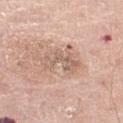Findings:
* notes — no biopsy performed (imaged during a skin exam)
* lighting — white-light
* acquisition — ~15 mm tile from a whole-body skin photo
* patient — male, aged approximately 80
* anatomic site — the left thigh